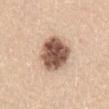<tbp_lesion>
  <biopsy_status>not biopsied; imaged during a skin examination</biopsy_status>
  <automated_metrics>
    <cielab_L>54</cielab_L>
    <cielab_a>19</cielab_a>
    <cielab_b>28</cielab_b>
    <vs_skin_darker_L>21.0</vs_skin_darker_L>
    <vs_skin_contrast_norm>13.0</vs_skin_contrast_norm>
    <color_variation_0_10>5.5</color_variation_0_10>
    <lesion_detection_confidence_0_100>100</lesion_detection_confidence_0_100>
  </automated_metrics>
  <image>
    <source>total-body photography crop</source>
    <field_of_view_mm>15</field_of_view_mm>
  </image>
  <patient>
    <sex>female</sex>
    <age_approx>65</age_approx>
  </patient>
  <site>lower back</site>
</tbp_lesion>